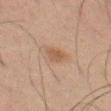{
  "biopsy_status": "not biopsied; imaged during a skin examination",
  "image": {
    "source": "total-body photography crop",
    "field_of_view_mm": 15
  },
  "patient": {
    "sex": "male",
    "age_approx": 65
  },
  "lighting": "cross-polarized",
  "lesion_size": {
    "long_diameter_mm_approx": 2.5
  },
  "automated_metrics": {
    "cielab_L": 44,
    "cielab_a": 16,
    "cielab_b": 27,
    "vs_skin_darker_L": 7.0,
    "vs_skin_contrast_norm": 6.5
  },
  "site": "left thigh"
}